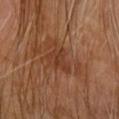Assessment: Imaged during a routine full-body skin examination; the lesion was not biopsied and no histopathology is available. Image and clinical context: Located on the right upper arm. The subject is a male roughly 60 years of age. A lesion tile, about 15 mm wide, cut from a 3D total-body photograph. Approximately 3 mm at its widest. Captured under cross-polarized illumination.